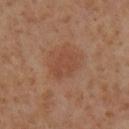Part of a total-body skin-imaging series; this lesion was reviewed on a skin check and was not flagged for biopsy. A female subject aged 53–57. A 15 mm crop from a total-body photograph taken for skin-cancer surveillance. Longest diameter approximately 3.5 mm. The lesion is on the left lower leg.